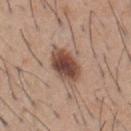notes: catalogued during a skin exam; not biopsied | patient: male, aged 58 to 62 | TBP lesion metrics: a shape eccentricity near 0.75; a lesion color around L≈46 a*≈20 b*≈26 in CIELAB, a lesion–skin lightness drop of about 16, and a lesion-to-skin contrast of about 11.5 (normalized; higher = more distinct); lesion-presence confidence of about 100/100 | diameter: about 4.5 mm | tile lighting: white-light | acquisition: 15 mm crop, total-body photography | body site: the chest.